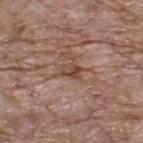The lesion was tiled from a total-body skin photograph and was not biopsied. A male subject, aged 68 to 72. A 15 mm close-up extracted from a 3D total-body photography capture. Captured under white-light illumination. The lesion-visualizer software estimated a mean CIELAB color near L≈46 a*≈20 b*≈26 and a lesion-to-skin contrast of about 7 (normalized; higher = more distinct). And it measured border irregularity of about 2.5 on a 0–10 scale and a within-lesion color-variation index near 5.5/10. And it measured a nevus-likeness score of about 0/100. On the upper back.